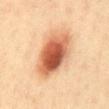biopsy status = no biopsy performed (imaged during a skin exam)
patient = male, about 40 years old
image source = ~15 mm tile from a whole-body skin photo
location = the abdomen
lesion diameter = ≈7 mm